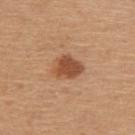Located on the upper back.
A female patient, aged around 45.
The lesion's longest dimension is about 3.5 mm.
The total-body-photography lesion software estimated an eccentricity of roughly 0.55 and a shape-asymmetry score of about 0.2 (0 = symmetric). The software also gave a lesion color around L≈51 a*≈23 b*≈35 in CIELAB. The software also gave a color-variation rating of about 3/10 and a peripheral color-asymmetry measure near 1.
A roughly 15 mm field-of-view crop from a total-body skin photograph.